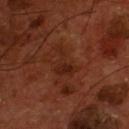Assessment: Recorded during total-body skin imaging; not selected for excision or biopsy. Acquisition and patient details: The lesion-visualizer software estimated a lesion area of about 6 mm², an eccentricity of roughly 0.75, and a symmetry-axis asymmetry near 0.7. And it measured a mean CIELAB color near L≈22 a*≈22 b*≈26, roughly 5 lightness units darker than nearby skin, and a normalized border contrast of about 6. The analysis additionally found a nevus-likeness score of about 0/100 and lesion-presence confidence of about 100/100. A male patient, roughly 55 years of age. The lesion is located on the chest. A 15 mm crop from a total-body photograph taken for skin-cancer surveillance.